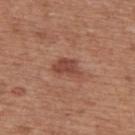No biopsy was performed on this lesion — it was imaged during a full skin examination and was not determined to be concerning. Longest diameter approximately 3.5 mm. A lesion tile, about 15 mm wide, cut from a 3D total-body photograph. The subject is a male about 65 years old. From the upper back.